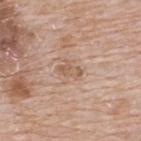{"biopsy_status": "not biopsied; imaged during a skin examination", "image": {"source": "total-body photography crop", "field_of_view_mm": 15}, "lesion_size": {"long_diameter_mm_approx": 3.0}, "lighting": "white-light", "site": "upper back", "patient": {"sex": "male", "age_approx": 65}}